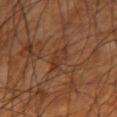| key | value |
|---|---|
| workup | imaged on a skin check; not biopsied |
| site | the left upper arm |
| subject | male, in their mid- to late 60s |
| image source | ~15 mm tile from a whole-body skin photo |
| illumination | cross-polarized |
| lesion size | about 3 mm |
| TBP lesion metrics | an area of roughly 5 mm² and a shape eccentricity near 0.65 |